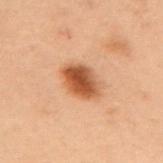Clinical impression:
Part of a total-body skin-imaging series; this lesion was reviewed on a skin check and was not flagged for biopsy.
Clinical summary:
The lesion's longest dimension is about 4.5 mm. The lesion is on the upper back. The patient is a female in their mid- to late 50s. Captured under cross-polarized illumination. Cropped from a total-body skin-imaging series; the visible field is about 15 mm.